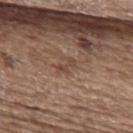biopsy_status: not biopsied; imaged during a skin examination
site: upper back
image:
  source: total-body photography crop
  field_of_view_mm: 15
patient:
  sex: female
  age_approx: 65